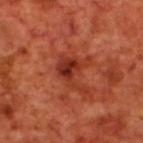The lesion was tiled from a total-body skin photograph and was not biopsied. Imaged with cross-polarized lighting. The recorded lesion diameter is about 5 mm. The subject is a male aged 68–72. From the back. A 15 mm close-up extracted from a 3D total-body photography capture. Automated image analysis of the tile measured an outline eccentricity of about 0.8 (0 = round, 1 = elongated) and a shape-asymmetry score of about 0.55 (0 = symmetric). The software also gave a border-irregularity index near 7/10, a color-variation rating of about 5/10, and peripheral color asymmetry of about 2.